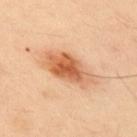follow-up: no biopsy performed (imaged during a skin exam) | patient: male, roughly 50 years of age | body site: the upper back | acquisition: 15 mm crop, total-body photography.